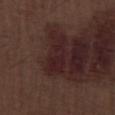Notes:
- follow-up · catalogued during a skin exam; not biopsied
- subject · male, approximately 70 years of age
- lesion diameter · about 5.5 mm
- image · ~15 mm crop, total-body skin-cancer survey
- TBP lesion metrics · an area of roughly 8 mm² and a symmetry-axis asymmetry near 0.45; a lesion-detection confidence of about 80/100
- body site · the right lower leg